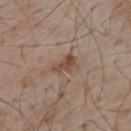workup — imaged on a skin check; not biopsied
illumination — white-light illumination
subject — male, in their mid-50s
body site — the upper back
image-analysis metrics — a lesion color around L≈48 a*≈17 b*≈28 in CIELAB and a lesion-to-skin contrast of about 7.5 (normalized; higher = more distinct); a border-irregularity index near 3.5/10 and a color-variation rating of about 4/10; an automated nevus-likeness rating near 10 out of 100 and lesion-presence confidence of about 100/100
imaging modality — ~15 mm crop, total-body skin-cancer survey
lesion size — ≈3 mm